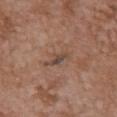{
  "lighting": "white-light",
  "patient": {
    "sex": "female",
    "age_approx": 65
  },
  "lesion_size": {
    "long_diameter_mm_approx": 3.0
  },
  "site": "chest",
  "image": {
    "source": "total-body photography crop",
    "field_of_view_mm": 15
  }
}